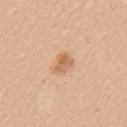biopsy_status: not biopsied; imaged during a skin examination
lesion_size:
  long_diameter_mm_approx: 2.5
lighting: white-light
image:
  source: total-body photography crop
  field_of_view_mm: 15
automated_metrics:
  cielab_L: 64
  cielab_a: 21
  cielab_b: 37
  vs_skin_darker_L: 10.0
site: left upper arm
patient:
  sex: female
  age_approx: 45